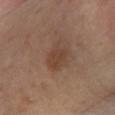The lesion was tiled from a total-body skin photograph and was not biopsied.